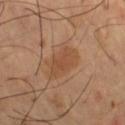Clinical impression: This lesion was catalogued during total-body skin photography and was not selected for biopsy.